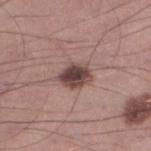<lesion>
  <biopsy_status>not biopsied; imaged during a skin examination</biopsy_status>
  <patient>
    <sex>male</sex>
    <age_approx>35</age_approx>
  </patient>
  <image>
    <source>total-body photography crop</source>
    <field_of_view_mm>15</field_of_view_mm>
  </image>
  <site>left lower leg</site>
  <lighting>white-light</lighting>
  <lesion_size>
    <long_diameter_mm_approx>3.5</long_diameter_mm_approx>
  </lesion_size>
</lesion>